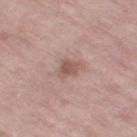follow-up = total-body-photography surveillance lesion; no biopsy
subject = female, aged 68 to 72
image source = ~15 mm crop, total-body skin-cancer survey
size = about 3 mm
body site = the right thigh